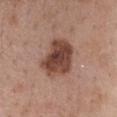Clinical impression: Part of a total-body skin-imaging series; this lesion was reviewed on a skin check and was not flagged for biopsy. Clinical summary: The patient is a female aged around 45. Imaged with white-light lighting. A 15 mm close-up extracted from a 3D total-body photography capture. The lesion-visualizer software estimated a lesion area of about 16 mm² and a shape-asymmetry score of about 0.2 (0 = symmetric). From the chest.